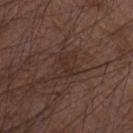| field | value |
|---|---|
| follow-up | catalogued during a skin exam; not biopsied |
| body site | the left forearm |
| subject | male, aged 48 to 52 |
| lesion size | ≈2.5 mm |
| acquisition | ~15 mm crop, total-body skin-cancer survey |
| lighting | white-light illumination |